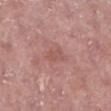Clinical impression: The lesion was photographed on a routine skin check and not biopsied; there is no pathology result. Clinical summary: A male subject, in their mid-50s. A lesion tile, about 15 mm wide, cut from a 3D total-body photograph. The lesion is on the leg.